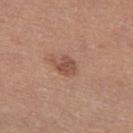Assessment:
Part of a total-body skin-imaging series; this lesion was reviewed on a skin check and was not flagged for biopsy.
Acquisition and patient details:
A 15 mm close-up extracted from a 3D total-body photography capture. The subject is a female about 35 years old. Imaged with white-light lighting. Measured at roughly 3 mm in maximum diameter. Located on the left thigh.